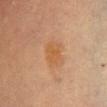Case summary:
– biopsy status · catalogued during a skin exam; not biopsied
– site · the chest
– image source · ~15 mm crop, total-body skin-cancer survey
– lighting · cross-polarized
– patient · female, approximately 60 years of age
– diameter · ~3 mm (longest diameter)
– automated lesion analysis · an automated nevus-likeness rating near 10 out of 100